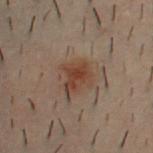notes = total-body-photography surveillance lesion; no biopsy
lesion diameter = about 3.5 mm
location = the chest
tile lighting = cross-polarized
patient = male, aged approximately 30
imaging modality = total-body-photography crop, ~15 mm field of view